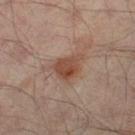patient: male, aged approximately 65 | acquisition: total-body-photography crop, ~15 mm field of view | anatomic site: the right thigh | lesion size: ≈4 mm.